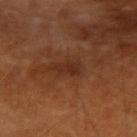Q: Is there a histopathology result?
A: no biopsy performed (imaged during a skin exam)
Q: What did automated image analysis measure?
A: a border-irregularity index near 3.5/10, internal color variation of about 2 on a 0–10 scale, and a peripheral color-asymmetry measure near 0.5; a classifier nevus-likeness of about 0/100 and lesion-presence confidence of about 100/100
Q: What is the anatomic site?
A: the right upper arm
Q: What are the patient's age and sex?
A: male, aged approximately 60
Q: What is the imaging modality?
A: total-body-photography crop, ~15 mm field of view
Q: Lesion size?
A: about 3 mm
Q: Illumination type?
A: cross-polarized